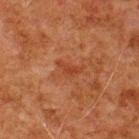The lesion was photographed on a routine skin check and not biopsied; there is no pathology result. Captured under cross-polarized illumination. A lesion tile, about 15 mm wide, cut from a 3D total-body photograph. On the upper back. Longest diameter approximately 3 mm. The subject is a male approximately 80 years of age. Automated image analysis of the tile measured an eccentricity of roughly 0.85 and two-axis asymmetry of about 0.5. And it measured an average lesion color of about L≈34 a*≈25 b*≈30 (CIELAB), a lesion–skin lightness drop of about 5, and a normalized lesion–skin contrast near 5.5.